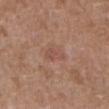| key | value |
|---|---|
| body site | the abdomen |
| lesion size | about 3 mm |
| image source | ~15 mm tile from a whole-body skin photo |
| subject | male, in their mid-70s |